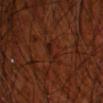biopsy status: imaged on a skin check; not biopsied
automated lesion analysis: a border-irregularity index near 5/10, a within-lesion color-variation index near 0.5/10, and peripheral color asymmetry of about 0
lesion size: ~3 mm (longest diameter)
image: total-body-photography crop, ~15 mm field of view
location: the left forearm
patient: male, about 70 years old
tile lighting: cross-polarized